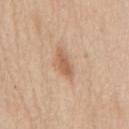workup = total-body-photography surveillance lesion; no biopsy
subject = male, roughly 60 years of age
acquisition = ~15 mm crop, total-body skin-cancer survey
anatomic site = the mid back